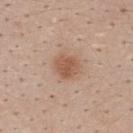Notes:
– workup: no biopsy performed (imaged during a skin exam)
– site: the back
– image source: 15 mm crop, total-body photography
– lesion size: about 3 mm
– automated metrics: a footprint of about 7 mm², a shape eccentricity near 0.45, and two-axis asymmetry of about 0.2
– illumination: white-light illumination
– patient: female, in their mid- to late 20s